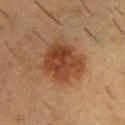follow-up = imaged on a skin check; not biopsied | subject = male, aged 53–57 | anatomic site = the back | image = 15 mm crop, total-body photography.